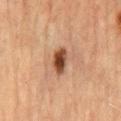The lesion was tiled from a total-body skin photograph and was not biopsied. A male subject in their mid- to late 60s. The lesion is on the abdomen. Cropped from a whole-body photographic skin survey; the tile spans about 15 mm. Automated image analysis of the tile measured a border-irregularity index near 2/10, internal color variation of about 4.5 on a 0–10 scale, and peripheral color asymmetry of about 1. Longest diameter approximately 3.5 mm.